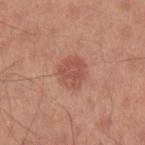Q: Was a biopsy performed?
A: imaged on a skin check; not biopsied
Q: What is the anatomic site?
A: the left upper arm
Q: What kind of image is this?
A: ~15 mm tile from a whole-body skin photo
Q: Automated lesion metrics?
A: an outline eccentricity of about 0.45 (0 = round, 1 = elongated) and two-axis asymmetry of about 0.15; a lesion-to-skin contrast of about 6 (normalized; higher = more distinct); a border-irregularity rating of about 2/10 and a color-variation rating of about 2/10
Q: Who is the patient?
A: male, about 30 years old